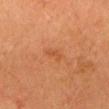Imaged during a routine full-body skin examination; the lesion was not biopsied and no histopathology is available.
Longest diameter approximately 2.5 mm.
This is a cross-polarized tile.
On the head or neck.
The patient is a female roughly 55 years of age.
A region of skin cropped from a whole-body photographic capture, roughly 15 mm wide.